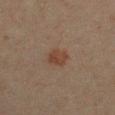Part of a total-body skin-imaging series; this lesion was reviewed on a skin check and was not flagged for biopsy. A female patient, aged 38 to 42. The recorded lesion diameter is about 2.5 mm. On the chest. A region of skin cropped from a whole-body photographic capture, roughly 15 mm wide. This is a cross-polarized tile. Automated image analysis of the tile measured a lesion color around L≈34 a*≈16 b*≈24 in CIELAB and a lesion-to-skin contrast of about 7 (normalized; higher = more distinct). And it measured a nevus-likeness score of about 95/100 and lesion-presence confidence of about 100/100.